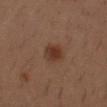Impression:
No biopsy was performed on this lesion — it was imaged during a full skin examination and was not determined to be concerning.
Context:
The subject is a male about 30 years old. On the arm. A 15 mm close-up extracted from a 3D total-body photography capture.